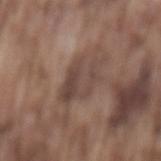The lesion was photographed on a routine skin check and not biopsied; there is no pathology result.
Captured under white-light illumination.
A 15 mm crop from a total-body photograph taken for skin-cancer surveillance.
The subject is a male aged approximately 75.
Located on the mid back.